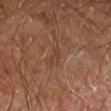Clinical impression: Imaged during a routine full-body skin examination; the lesion was not biopsied and no histopathology is available. Clinical summary: The lesion is located on the right leg. About 2.5 mm across. A 15 mm close-up tile from a total-body photography series done for melanoma screening. Captured under cross-polarized illumination. Automated tile analysis of the lesion measured an outline eccentricity of about 0.95 (0 = round, 1 = elongated) and a shape-asymmetry score of about 0.45 (0 = symmetric). It also reported border irregularity of about 5.5 on a 0–10 scale and a color-variation rating of about 0/10. A male patient approximately 60 years of age.